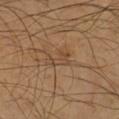<case>
<biopsy_status>not biopsied; imaged during a skin examination</biopsy_status>
<patient>
  <sex>male</sex>
  <age_approx>65</age_approx>
</patient>
<site>left forearm</site>
<image>
  <source>total-body photography crop</source>
  <field_of_view_mm>15</field_of_view_mm>
</image>
</case>